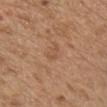Case summary:
• anatomic site — the chest
• image-analysis metrics — a lesion area of about 2.5 mm² and an eccentricity of roughly 0.9; a mean CIELAB color near L≈52 a*≈20 b*≈33 and a normalized lesion–skin contrast near 4.5
• tile lighting — white-light
• patient — male, aged approximately 70
• image source — ~15 mm tile from a whole-body skin photo
• size — ~2.5 mm (longest diameter)